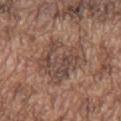No biopsy was performed on this lesion — it was imaged during a full skin examination and was not determined to be concerning.